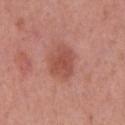The lesion was tiled from a total-body skin photograph and was not biopsied.